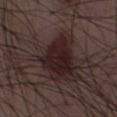{
  "biopsy_status": "not biopsied; imaged during a skin examination",
  "lighting": "white-light",
  "lesion_size": {
    "long_diameter_mm_approx": 5.0
  },
  "image": {
    "source": "total-body photography crop",
    "field_of_view_mm": 15
  },
  "patient": {
    "sex": "male",
    "age_approx": 50
  },
  "automated_metrics": {
    "area_mm2_approx": 14.0,
    "shape_asymmetry": 0.35,
    "cielab_L": 19,
    "cielab_a": 15,
    "cielab_b": 15,
    "vs_skin_darker_L": 10.0,
    "vs_skin_contrast_norm": 11.5,
    "nevus_likeness_0_100": 80,
    "lesion_detection_confidence_0_100": 100
  }
}